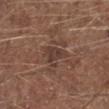notes=total-body-photography surveillance lesion; no biopsy
patient=male, aged 73–77
body site=the left lower leg
acquisition=15 mm crop, total-body photography
size=about 3.5 mm